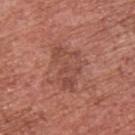follow-up = no biopsy performed (imaged during a skin exam) | location = the chest | tile lighting = white-light | image = total-body-photography crop, ~15 mm field of view | lesion size = ~5.5 mm (longest diameter) | subject = male, about 45 years old.